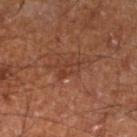This lesion was catalogued during total-body skin photography and was not selected for biopsy.
Automated tile analysis of the lesion measured a lesion area of about 3.5 mm², an outline eccentricity of about 0.85 (0 = round, 1 = elongated), and a symmetry-axis asymmetry near 0.45. It also reported an average lesion color of about L≈37 a*≈23 b*≈29 (CIELAB), a lesion–skin lightness drop of about 5, and a lesion-to-skin contrast of about 5 (normalized; higher = more distinct). The analysis additionally found a border-irregularity index near 4.5/10, internal color variation of about 2.5 on a 0–10 scale, and a peripheral color-asymmetry measure near 1.
A male subject, aged around 60.
This is a cross-polarized tile.
On the left lower leg.
A roughly 15 mm field-of-view crop from a total-body skin photograph.